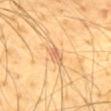{"biopsy_status": "not biopsied; imaged during a skin examination", "patient": {"sex": "male", "age_approx": 65}, "lighting": "cross-polarized", "site": "mid back", "lesion_size": {"long_diameter_mm_approx": 2.5}, "automated_metrics": {"area_mm2_approx": 3.5, "eccentricity": 0.7, "shape_asymmetry": 0.25, "border_irregularity_0_10": 2.0, "color_variation_0_10": 2.0, "peripheral_color_asymmetry": 0.5, "nevus_likeness_0_100": 0, "lesion_detection_confidence_0_100": 90}, "image": {"source": "total-body photography crop", "field_of_view_mm": 15}}